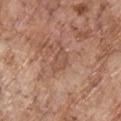Clinical impression:
Part of a total-body skin-imaging series; this lesion was reviewed on a skin check and was not flagged for biopsy.
Acquisition and patient details:
Imaged with white-light lighting. A 15 mm close-up extracted from a 3D total-body photography capture. The patient is a male aged 68–72. An algorithmic analysis of the crop reported a footprint of about 4 mm² and two-axis asymmetry of about 0.4. The software also gave about 7 CIELAB-L* units darker than the surrounding skin. It also reported a border-irregularity index near 3.5/10, a color-variation rating of about 1.5/10, and a peripheral color-asymmetry measure near 0.5. Located on the chest.